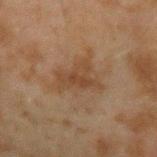{"site": "left forearm", "image": {"source": "total-body photography crop", "field_of_view_mm": 15}, "automated_metrics": {"area_mm2_approx": 9.0, "eccentricity": 0.75, "shape_asymmetry": 0.55, "cielab_L": 35, "cielab_a": 15, "cielab_b": 26, "vs_skin_darker_L": 6.0, "vs_skin_contrast_norm": 6.5}, "patient": {"sex": "male", "age_approx": 45}, "lesion_size": {"long_diameter_mm_approx": 4.5}, "lighting": "cross-polarized"}